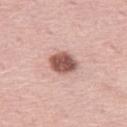Recorded during total-body skin imaging; not selected for excision or biopsy.